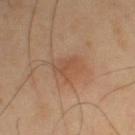This lesion was catalogued during total-body skin photography and was not selected for biopsy.
A male subject, in their mid- to late 40s.
The tile uses cross-polarized illumination.
The recorded lesion diameter is about 3.5 mm.
Automated image analysis of the tile measured an eccentricity of roughly 0.65 and two-axis asymmetry of about 0.3. The software also gave an automated nevus-likeness rating near 15 out of 100 and a lesion-detection confidence of about 100/100.
A region of skin cropped from a whole-body photographic capture, roughly 15 mm wide.
From the right upper arm.